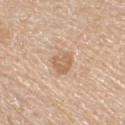* notes — no biopsy performed (imaged during a skin exam)
* image — ~15 mm tile from a whole-body skin photo
* tile lighting — white-light illumination
* site — the left lower leg
* size — about 3 mm
* patient — female, aged around 70